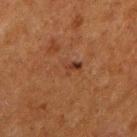Recorded during total-body skin imaging; not selected for excision or biopsy. The lesion-visualizer software estimated an area of roughly 5.5 mm². The software also gave a lesion–skin lightness drop of about 5 and a lesion-to-skin contrast of about 4.5 (normalized; higher = more distinct). The analysis additionally found a border-irregularity rating of about 3.5/10, a within-lesion color-variation index near 7/10, and radial color variation of about 3. It also reported an automated nevus-likeness rating near 0 out of 100 and a lesion-detection confidence of about 100/100. The lesion is located on the right upper arm. About 3.5 mm across. A 15 mm close-up extracted from a 3D total-body photography capture. A female patient aged 48–52.